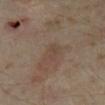Impression: Imaged during a routine full-body skin examination; the lesion was not biopsied and no histopathology is available. Acquisition and patient details: About 3 mm across. The tile uses cross-polarized illumination. A female subject aged around 35. The lesion is on the leg. A lesion tile, about 15 mm wide, cut from a 3D total-body photograph.